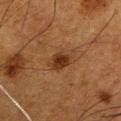<lesion>
  <biopsy_status>not biopsied; imaged during a skin examination</biopsy_status>
  <site>left upper arm</site>
  <image>
    <source>total-body photography crop</source>
    <field_of_view_mm>15</field_of_view_mm>
  </image>
  <patient>
    <sex>male</sex>
    <age_approx>50</age_approx>
  </patient>
  <lighting>cross-polarized</lighting>
  <automated_metrics>
    <border_irregularity_0_10>3.0</border_irregularity_0_10>
    <color_variation_0_10>3.0</color_variation_0_10>
    <peripheral_color_asymmetry>1.0</peripheral_color_asymmetry>
  </automated_metrics>
</lesion>